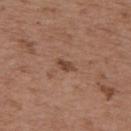The total-body-photography lesion software estimated a lesion area of about 2.5 mm², a shape eccentricity near 0.9, and a symmetry-axis asymmetry near 0.3. And it measured a mean CIELAB color near L≈44 a*≈19 b*≈28.
Captured under white-light illumination.
Approximately 2.5 mm at its widest.
A female patient aged approximately 65.
The lesion is on the upper back.
A close-up tile cropped from a whole-body skin photograph, about 15 mm across.